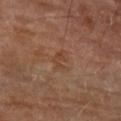  biopsy_status: not biopsied; imaged during a skin examination
  lesion_size:
    long_diameter_mm_approx: 2.5
  patient:
    sex: male
    age_approx: 70
  lighting: cross-polarized
  site: right lower leg
  image:
    source: total-body photography crop
    field_of_view_mm: 15